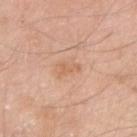follow-up: total-body-photography surveillance lesion; no biopsy | patient: male, aged 58 to 62 | acquisition: ~15 mm tile from a whole-body skin photo | TBP lesion metrics: a mean CIELAB color near L≈63 a*≈22 b*≈34, roughly 7 lightness units darker than nearby skin, and a lesion-to-skin contrast of about 5 (normalized; higher = more distinct); a detector confidence of about 100 out of 100 that the crop contains a lesion | location: the right upper arm | lesion diameter: ~2.5 mm (longest diameter) | lighting: white-light.